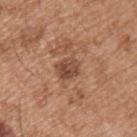{"biopsy_status": "not biopsied; imaged during a skin examination", "lighting": "white-light", "lesion_size": {"long_diameter_mm_approx": 2.5}, "site": "left upper arm", "patient": {"sex": "male", "age_approx": 55}, "image": {"source": "total-body photography crop", "field_of_view_mm": 15}}